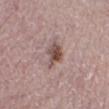Impression: Imaged during a routine full-body skin examination; the lesion was not biopsied and no histopathology is available. Clinical summary: The lesion is on the right lower leg. A 15 mm close-up tile from a total-body photography series done for melanoma screening. Longest diameter approximately 3 mm. This is a white-light tile. Automated image analysis of the tile measured an outline eccentricity of about 0.5 (0 = round, 1 = elongated) and two-axis asymmetry of about 0.3. The analysis additionally found a lesion color around L≈49 a*≈18 b*≈21 in CIELAB, roughly 13 lightness units darker than nearby skin, and a normalized lesion–skin contrast near 9. A female patient aged 48 to 52.